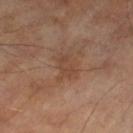A male patient roughly 70 years of age.
Cropped from a whole-body photographic skin survey; the tile spans about 15 mm.
This is a cross-polarized tile.
The recorded lesion diameter is about 2.5 mm.
The lesion-visualizer software estimated a lesion color around L≈42 a*≈19 b*≈28 in CIELAB and roughly 6 lightness units darker than nearby skin. It also reported border irregularity of about 6 on a 0–10 scale and a color-variation rating of about 0/10.
From the left thigh.